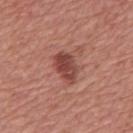Assessment: Part of a total-body skin-imaging series; this lesion was reviewed on a skin check and was not flagged for biopsy. Background: A male subject, approximately 65 years of age. This is a white-light tile. The lesion is located on the back. Measured at roughly 3.5 mm in maximum diameter. An algorithmic analysis of the crop reported a lesion area of about 7.5 mm², a shape eccentricity near 0.75, and a shape-asymmetry score of about 0.2 (0 = symmetric). The software also gave about 12 CIELAB-L* units darker than the surrounding skin and a normalized lesion–skin contrast near 9. The analysis additionally found border irregularity of about 2.5 on a 0–10 scale, a within-lesion color-variation index near 4/10, and a peripheral color-asymmetry measure near 1.5. A close-up tile cropped from a whole-body skin photograph, about 15 mm across.